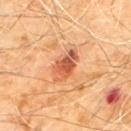Assessment:
Imaged during a routine full-body skin examination; the lesion was not biopsied and no histopathology is available.
Context:
The lesion is on the chest. A male patient aged 58–62. Captured under cross-polarized illumination. A 15 mm close-up extracted from a 3D total-body photography capture. The total-body-photography lesion software estimated a border-irregularity index near 2.5/10 and a color-variation rating of about 6.5/10. Approximately 4.5 mm at its widest.